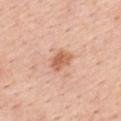Imaged during a routine full-body skin examination; the lesion was not biopsied and no histopathology is available. The lesion is on the back. A close-up tile cropped from a whole-body skin photograph, about 15 mm across. A female patient aged 43 to 47. Approximately 3 mm at its widest.